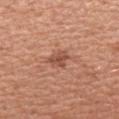Assessment: Imaged during a routine full-body skin examination; the lesion was not biopsied and no histopathology is available. Context: Automated image analysis of the tile measured a footprint of about 4.5 mm², an eccentricity of roughly 0.65, and two-axis asymmetry of about 0.2. It also reported peripheral color asymmetry of about 1. The patient is a female about 50 years old. A close-up tile cropped from a whole-body skin photograph, about 15 mm across. From the arm.